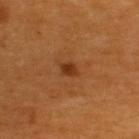Q: Was a biopsy performed?
A: total-body-photography surveillance lesion; no biopsy
Q: What did automated image analysis measure?
A: an eccentricity of roughly 0.65; a lesion color around L≈34 a*≈25 b*≈36 in CIELAB, about 9 CIELAB-L* units darker than the surrounding skin, and a normalized lesion–skin contrast near 8.5; a color-variation rating of about 2/10 and peripheral color asymmetry of about 0.5; a detector confidence of about 100 out of 100 that the crop contains a lesion
Q: What is the lesion's diameter?
A: about 2 mm
Q: What lighting was used for the tile?
A: cross-polarized illumination
Q: Who is the patient?
A: male, aged around 60
Q: Lesion location?
A: the back
Q: What is the imaging modality?
A: ~15 mm crop, total-body skin-cancer survey